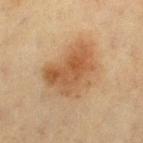Q: Was a biopsy performed?
A: catalogued during a skin exam; not biopsied
Q: What is the imaging modality?
A: ~15 mm crop, total-body skin-cancer survey
Q: How was the tile lit?
A: cross-polarized illumination
Q: What are the patient's age and sex?
A: female, aged 48 to 52
Q: What is the anatomic site?
A: the left thigh
Q: What is the lesion's diameter?
A: about 6.5 mm
Q: Automated lesion metrics?
A: a footprint of about 24 mm², an eccentricity of roughly 0.7, and two-axis asymmetry of about 0.25; an average lesion color of about L≈46 a*≈17 b*≈32 (CIELAB) and roughly 9 lightness units darker than nearby skin; border irregularity of about 3 on a 0–10 scale and a within-lesion color-variation index near 5/10